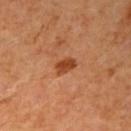Impression:
Recorded during total-body skin imaging; not selected for excision or biopsy.
Clinical summary:
A female patient approximately 40 years of age. Located on the right upper arm. Longest diameter approximately 2.5 mm. A lesion tile, about 15 mm wide, cut from a 3D total-body photograph.